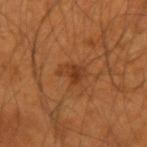Clinical impression: The lesion was tiled from a total-body skin photograph and was not biopsied. Clinical summary: This is a cross-polarized tile. A roughly 15 mm field-of-view crop from a total-body skin photograph. A male subject, in their mid- to late 50s. Located on the left forearm.